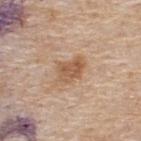This lesion was catalogued during total-body skin photography and was not selected for biopsy.
Imaged with white-light lighting.
The patient is a male aged 53–57.
A 15 mm close-up tile from a total-body photography series done for melanoma screening.
The lesion is on the back.